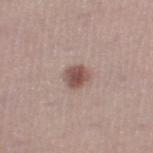biopsy status=total-body-photography surveillance lesion; no biopsy | location=the right thigh | acquisition=~15 mm crop, total-body skin-cancer survey | tile lighting=white-light | lesion diameter=about 2.5 mm | patient=male, aged approximately 55.